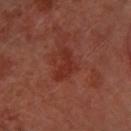Captured during whole-body skin photography for melanoma surveillance; the lesion was not biopsied. A female subject aged 63 to 67. The lesion is located on the left forearm. Automated image analysis of the tile measured a footprint of about 6 mm², an outline eccentricity of about 0.9 (0 = round, 1 = elongated), and a shape-asymmetry score of about 0.55 (0 = symmetric). It also reported an automated nevus-likeness rating near 0 out of 100 and a detector confidence of about 100 out of 100 that the crop contains a lesion. Longest diameter approximately 4.5 mm. Imaged with cross-polarized lighting. A close-up tile cropped from a whole-body skin photograph, about 15 mm across.